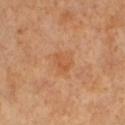biopsy_status: not biopsied; imaged during a skin examination
image:
  source: total-body photography crop
  field_of_view_mm: 15
site: left lower leg
patient:
  sex: female
  age_approx: 55
lesion_size:
  long_diameter_mm_approx: 2.5
lighting: cross-polarized